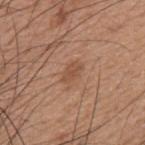Captured during whole-body skin photography for melanoma surveillance; the lesion was not biopsied. This image is a 15 mm lesion crop taken from a total-body photograph. From the back. A male subject, approximately 20 years of age. The lesion's longest dimension is about 2.5 mm.